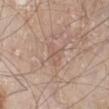No biopsy was performed on this lesion — it was imaged during a full skin examination and was not determined to be concerning. A male patient aged 58 to 62. A 15 mm close-up extracted from a 3D total-body photography capture. From the left lower leg. The lesion-visualizer software estimated a symmetry-axis asymmetry near 0.3. The software also gave a normalized border contrast of about 4. It also reported a nevus-likeness score of about 0/100. Captured under white-light illumination.